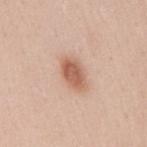Q: Was a biopsy performed?
A: catalogued during a skin exam; not biopsied
Q: How large is the lesion?
A: about 4 mm
Q: What is the anatomic site?
A: the mid back
Q: What kind of image is this?
A: 15 mm crop, total-body photography
Q: Patient demographics?
A: female, aged around 30